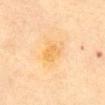| field | value |
|---|---|
| follow-up | total-body-photography surveillance lesion; no biopsy |
| body site | the front of the torso |
| diameter | about 2.5 mm |
| image | ~15 mm tile from a whole-body skin photo |
| patient | female, in their 70s |